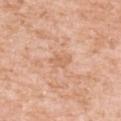Clinical impression: Recorded during total-body skin imaging; not selected for excision or biopsy. Context: A roughly 15 mm field-of-view crop from a total-body skin photograph. The lesion is located on the left upper arm. Automated tile analysis of the lesion measured a lesion area of about 3 mm², an outline eccentricity of about 0.85 (0 = round, 1 = elongated), and a shape-asymmetry score of about 0.4 (0 = symmetric). And it measured an average lesion color of about L≈64 a*≈22 b*≈34 (CIELAB), about 7 CIELAB-L* units darker than the surrounding skin, and a normalized border contrast of about 5. And it measured a border-irregularity rating of about 3.5/10, a within-lesion color-variation index near 1.5/10, and peripheral color asymmetry of about 0.5. The recorded lesion diameter is about 2.5 mm. The tile uses white-light illumination. The subject is a female approximately 40 years of age.